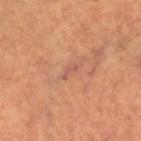Part of a total-body skin-imaging series; this lesion was reviewed on a skin check and was not flagged for biopsy. A 15 mm close-up extracted from a 3D total-body photography capture. A female patient, about 65 years old. About 2.5 mm across. The lesion is located on the right lower leg.